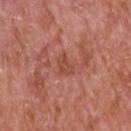workup: no biopsy performed (imaged during a skin exam)
image source: ~15 mm tile from a whole-body skin photo
subject: male, roughly 65 years of age
anatomic site: the upper back